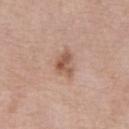Imaged during a routine full-body skin examination; the lesion was not biopsied and no histopathology is available. A male subject, approximately 75 years of age. A 15 mm close-up tile from a total-body photography series done for melanoma screening. On the chest.